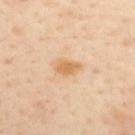biopsy status = imaged on a skin check; not biopsied | location = the upper back | diameter = ≈3 mm | imaging modality = total-body-photography crop, ~15 mm field of view | subject = female, in their 40s.